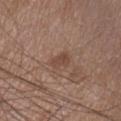Case summary:
• follow-up: no biopsy performed (imaged during a skin exam)
• anatomic site: the upper back
• tile lighting: white-light illumination
• patient: female, aged approximately 70
• TBP lesion metrics: a lesion color around L≈45 a*≈18 b*≈25 in CIELAB, a lesion–skin lightness drop of about 8, and a lesion-to-skin contrast of about 6.5 (normalized; higher = more distinct); a border-irregularity rating of about 3/10 and a color-variation rating of about 1.5/10; a nevus-likeness score of about 0/100 and lesion-presence confidence of about 100/100
• lesion diameter: ≈3 mm
• image: total-body-photography crop, ~15 mm field of view